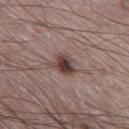This is a white-light tile.
The lesion is on the leg.
A male patient in their mid-70s.
The total-body-photography lesion software estimated a border-irregularity rating of about 2.5/10, internal color variation of about 8 on a 0–10 scale, and peripheral color asymmetry of about 2. And it measured a lesion-detection confidence of about 100/100.
A roughly 15 mm field-of-view crop from a total-body skin photograph.
The lesion's longest dimension is about 3.5 mm.